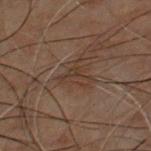{
  "biopsy_status": "not biopsied; imaged during a skin examination",
  "automated_metrics": {
    "area_mm2_approx": 6.5,
    "eccentricity": 0.7,
    "border_irregularity_0_10": 5.5,
    "peripheral_color_asymmetry": 0.5
  },
  "lighting": "cross-polarized",
  "lesion_size": {
    "long_diameter_mm_approx": 4.0
  },
  "patient": {
    "sex": "male",
    "age_approx": 50
  },
  "site": "front of the torso",
  "image": {
    "source": "total-body photography crop",
    "field_of_view_mm": 15
  }
}